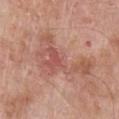A close-up tile cropped from a whole-body skin photograph, about 15 mm across.
The lesion is located on the chest.
A male subject, aged approximately 65.